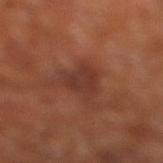follow-up: total-body-photography surveillance lesion; no biopsy
imaging modality: 15 mm crop, total-body photography
site: the right lower leg
patient: about 65 years old
image-analysis metrics: an area of roughly 8.5 mm² and an outline eccentricity of about 0.7 (0 = round, 1 = elongated); border irregularity of about 4 on a 0–10 scale, internal color variation of about 2 on a 0–10 scale, and a peripheral color-asymmetry measure near 0.5; an automated nevus-likeness rating near 0 out of 100 and lesion-presence confidence of about 100/100
tile lighting: cross-polarized illumination
lesion diameter: ~4.5 mm (longest diameter)